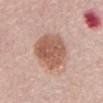<lesion>
  <biopsy_status>not biopsied; imaged during a skin examination</biopsy_status>
  <lighting>white-light</lighting>
  <image>
    <source>total-body photography crop</source>
    <field_of_view_mm>15</field_of_view_mm>
  </image>
  <site>abdomen</site>
  <lesion_size>
    <long_diameter_mm_approx>5.5</long_diameter_mm_approx>
  </lesion_size>
  <patient>
    <sex>female</sex>
    <age_approx>65</age_approx>
  </patient>
</lesion>